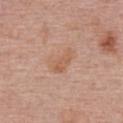{
  "biopsy_status": "not biopsied; imaged during a skin examination",
  "image": {
    "source": "total-body photography crop",
    "field_of_view_mm": 15
  },
  "patient": {
    "sex": "female",
    "age_approx": 65
  },
  "site": "upper back"
}